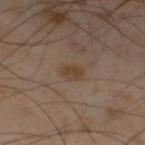{
  "biopsy_status": "not biopsied; imaged during a skin examination"
}